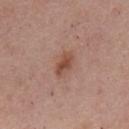Case summary:
– follow-up — total-body-photography surveillance lesion; no biopsy
– patient — male, about 55 years old
– image-analysis metrics — a lesion area of about 5 mm², a shape eccentricity near 0.85, and a symmetry-axis asymmetry near 0.2; a border-irregularity index near 2/10; a classifier nevus-likeness of about 75/100 and a lesion-detection confidence of about 100/100
– diameter — ≈3.5 mm
– tile lighting — white-light illumination
– anatomic site — the back
– acquisition — ~15 mm crop, total-body skin-cancer survey